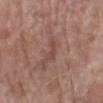| field | value |
|---|---|
| biopsy status | total-body-photography surveillance lesion; no biopsy |
| lighting | white-light |
| automated lesion analysis | an area of roughly 3 mm² and a symmetry-axis asymmetry near 0.4; an average lesion color of about L≈46 a*≈21 b*≈24 (CIELAB) and a lesion-to-skin contrast of about 5.5 (normalized; higher = more distinct); a classifier nevus-likeness of about 0/100 and a lesion-detection confidence of about 55/100 |
| patient | female, aged approximately 70 |
| size | ≈3 mm |
| body site | the arm |
| image source | 15 mm crop, total-body photography |